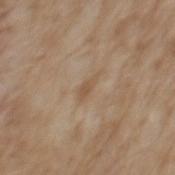| field | value |
|---|---|
| anatomic site | the back |
| patient | male, about 70 years old |
| acquisition | 15 mm crop, total-body photography |
| lighting | white-light illumination |